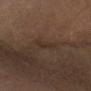Imaged during a routine full-body skin examination; the lesion was not biopsied and no histopathology is available. On the arm. A female subject aged 48 to 52. A region of skin cropped from a whole-body photographic capture, roughly 15 mm wide.